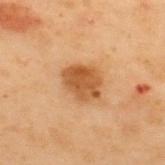notes: no biopsy performed (imaged during a skin exam)
diameter: ~4 mm (longest diameter)
TBP lesion metrics: a lesion color around L≈44 a*≈21 b*≈35 in CIELAB, roughly 11 lightness units darker than nearby skin, and a lesion-to-skin contrast of about 9 (normalized; higher = more distinct)
imaging modality: ~15 mm tile from a whole-body skin photo
lighting: cross-polarized illumination
location: the upper back
subject: male, aged 53 to 57